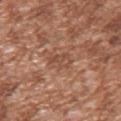{"biopsy_status": "not biopsied; imaged during a skin examination", "patient": {"sex": "male", "age_approx": 45}, "automated_metrics": {"area_mm2_approx": 4.0, "shape_asymmetry": 0.4, "border_irregularity_0_10": 4.0, "color_variation_0_10": 2.0, "peripheral_color_asymmetry": 0.5}, "lighting": "white-light", "lesion_size": {"long_diameter_mm_approx": 3.0}, "image": {"source": "total-body photography crop", "field_of_view_mm": 15}, "site": "arm"}